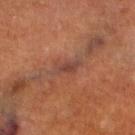| key | value |
|---|---|
| workup | total-body-photography surveillance lesion; no biopsy |